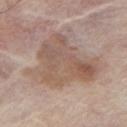body site: the chest; patient: male, aged 78–82; lesion diameter: ≈8 mm; image source: 15 mm crop, total-body photography.